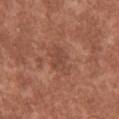notes = catalogued during a skin exam; not biopsied | diameter = ≈4 mm | tile lighting = white-light | body site = the upper back | image source = ~15 mm crop, total-body skin-cancer survey | subject = male, approximately 45 years of age.